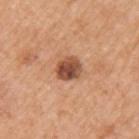The lesion was photographed on a routine skin check and not biopsied; there is no pathology result.
A female patient, aged approximately 55.
Imaged with white-light lighting.
A region of skin cropped from a whole-body photographic capture, roughly 15 mm wide.
The lesion is located on the left upper arm.
Longest diameter approximately 3 mm.